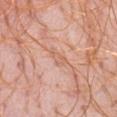{"biopsy_status": "not biopsied; imaged during a skin examination", "lesion_size": {"long_diameter_mm_approx": 2.5}, "lighting": "white-light", "automated_metrics": {"area_mm2_approx": 3.0, "eccentricity": 0.85, "cielab_L": 64, "cielab_a": 23, "cielab_b": 32, "vs_skin_darker_L": 6.0, "vs_skin_contrast_norm": 4.5}, "site": "abdomen", "image": {"source": "total-body photography crop", "field_of_view_mm": 15}, "patient": {"sex": "female", "age_approx": 45}}